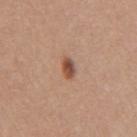Context: A region of skin cropped from a whole-body photographic capture, roughly 15 mm wide. The patient is a female aged 28 to 32. The lesion's longest dimension is about 2.5 mm. This is a white-light tile. Automated image analysis of the tile measured a footprint of about 3.5 mm², a shape eccentricity near 0.8, and a symmetry-axis asymmetry near 0.15. The analysis additionally found a mean CIELAB color near L≈50 a*≈23 b*≈30, roughly 13 lightness units darker than nearby skin, and a normalized border contrast of about 9. Located on the right upper arm.